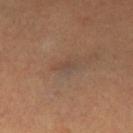The lesion was photographed on a routine skin check and not biopsied; there is no pathology result. A 15 mm close-up extracted from a 3D total-body photography capture. The lesion is on the left thigh. The subject is a female approximately 30 years of age. The total-body-photography lesion software estimated a footprint of about 3 mm², an eccentricity of roughly 0.85, and two-axis asymmetry of about 0.4. And it measured a border-irregularity rating of about 3.5/10, internal color variation of about 1.5 on a 0–10 scale, and peripheral color asymmetry of about 0.5. The tile uses cross-polarized illumination.